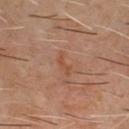The lesion was tiled from a total-body skin photograph and was not biopsied. The patient is a male roughly 60 years of age. The lesion is located on the chest. Imaged with cross-polarized lighting. About 3 mm across. Automated image analysis of the tile measured a footprint of about 2.5 mm² and a shape eccentricity near 0.95. The software also gave about 6 CIELAB-L* units darker than the surrounding skin and a lesion-to-skin contrast of about 5.5 (normalized; higher = more distinct). And it measured border irregularity of about 6 on a 0–10 scale, internal color variation of about 0 on a 0–10 scale, and peripheral color asymmetry of about 0. A 15 mm crop from a total-body photograph taken for skin-cancer surveillance.